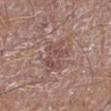Imaged during a routine full-body skin examination; the lesion was not biopsied and no histopathology is available. A male subject, aged around 70. A region of skin cropped from a whole-body photographic capture, roughly 15 mm wide. Imaged with white-light lighting. From the left lower leg.